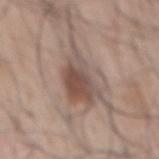Recorded during total-body skin imaging; not selected for excision or biopsy. This is a white-light tile. On the back. A male patient, aged approximately 70. The total-body-photography lesion software estimated an average lesion color of about L≈50 a*≈16 b*≈23 (CIELAB), roughly 11 lightness units darker than nearby skin, and a normalized border contrast of about 8. The analysis additionally found a border-irregularity index near 6/10, internal color variation of about 6.5 on a 0–10 scale, and a peripheral color-asymmetry measure near 2. A lesion tile, about 15 mm wide, cut from a 3D total-body photograph. Longest diameter approximately 7.5 mm.